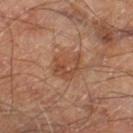notes: imaged on a skin check; not biopsied
illumination: cross-polarized illumination
size: ≈3.5 mm
image: total-body-photography crop, ~15 mm field of view
location: the right lower leg
patient: male, aged 68–72